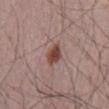biopsy status: no biopsy performed (imaged during a skin exam)
acquisition: ~15 mm tile from a whole-body skin photo
illumination: white-light
anatomic site: the mid back
subject: male, in their mid-60s
size: ≈2.5 mm